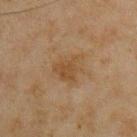Impression:
This lesion was catalogued during total-body skin photography and was not selected for biopsy.
Background:
A roughly 15 mm field-of-view crop from a total-body skin photograph. From the front of the torso. A male subject, approximately 45 years of age.